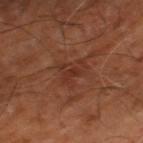The lesion was tiled from a total-body skin photograph and was not biopsied. The lesion is on the left thigh. This image is a 15 mm lesion crop taken from a total-body photograph. The patient is aged around 65.